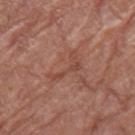Clinical impression:
The lesion was photographed on a routine skin check and not biopsied; there is no pathology result.
Acquisition and patient details:
This image is a 15 mm lesion crop taken from a total-body photograph. About 4 mm across. A female subject roughly 80 years of age. The lesion is on the right thigh. The total-body-photography lesion software estimated a footprint of about 4.5 mm², an outline eccentricity of about 0.9 (0 = round, 1 = elongated), and a shape-asymmetry score of about 0.75 (0 = symmetric). The software also gave a lesion color around L≈46 a*≈24 b*≈28 in CIELAB and a normalized border contrast of about 5.5. The analysis additionally found border irregularity of about 9 on a 0–10 scale and a color-variation rating of about 0/10. Imaged with white-light lighting.